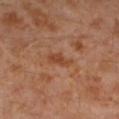Captured during whole-body skin photography for melanoma surveillance; the lesion was not biopsied. The patient is a male roughly 30 years of age. A roughly 15 mm field-of-view crop from a total-body skin photograph. The tile uses cross-polarized illumination. The total-body-photography lesion software estimated an average lesion color of about L≈43 a*≈23 b*≈33 (CIELAB), roughly 7 lightness units darker than nearby skin, and a normalized lesion–skin contrast near 6.5. It also reported peripheral color asymmetry of about 0.5. The lesion is located on the leg.